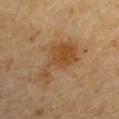| key | value |
|---|---|
| workup | no biopsy performed (imaged during a skin exam) |
| automated metrics | about 7 CIELAB-L* units darker than the surrounding skin; a border-irregularity index near 7.5/10, a color-variation rating of about 2.5/10, and radial color variation of about 0.5; a classifier nevus-likeness of about 95/100 |
| patient | male, roughly 65 years of age |
| body site | the right upper arm |
| tile lighting | cross-polarized illumination |
| image | total-body-photography crop, ~15 mm field of view |
| size | about 7 mm |